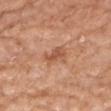Imaged during a routine full-body skin examination; the lesion was not biopsied and no histopathology is available.
The lesion's longest dimension is about 3.5 mm.
On the right upper arm.
Cropped from a whole-body photographic skin survey; the tile spans about 15 mm.
Imaged with white-light lighting.
The total-body-photography lesion software estimated a lesion area of about 5 mm² and a shape-asymmetry score of about 0.25 (0 = symmetric). It also reported a within-lesion color-variation index near 2/10. The analysis additionally found an automated nevus-likeness rating near 0 out of 100 and lesion-presence confidence of about 100/100.
A female subject, about 75 years old.